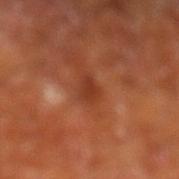Q: What kind of image is this?
A: 15 mm crop, total-body photography
Q: What are the patient's age and sex?
A: male, about 65 years old
Q: What is the anatomic site?
A: the left lower leg